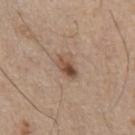Case summary:
– notes · total-body-photography surveillance lesion; no biopsy
– location · the chest
– lesion diameter · ~3 mm (longest diameter)
– patient · male, in their mid-70s
– automated lesion analysis · an area of roughly 4.5 mm², an eccentricity of roughly 0.85, and a shape-asymmetry score of about 0.3 (0 = symmetric); a lesion color around L≈49 a*≈17 b*≈28 in CIELAB and a normalized lesion–skin contrast near 9; a border-irregularity rating of about 3/10, internal color variation of about 8 on a 0–10 scale, and radial color variation of about 3
– image · ~15 mm tile from a whole-body skin photo